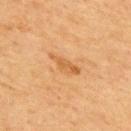Imaged during a routine full-body skin examination; the lesion was not biopsied and no histopathology is available.
Cropped from a whole-body photographic skin survey; the tile spans about 15 mm.
Imaged with cross-polarized lighting.
Automated tile analysis of the lesion measured a lesion color around L≈52 a*≈21 b*≈39 in CIELAB and a lesion-to-skin contrast of about 6 (normalized; higher = more distinct). It also reported border irregularity of about 4.5 on a 0–10 scale, a color-variation rating of about 2.5/10, and peripheral color asymmetry of about 0.5. It also reported a detector confidence of about 100 out of 100 that the crop contains a lesion.
From the upper back.
The lesion's longest dimension is about 4 mm.
The patient is a male in their mid- to late 60s.